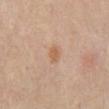Acquisition and patient details: A male patient aged 63 to 67. This image is a 15 mm lesion crop taken from a total-body photograph. On the abdomen. This is a white-light tile. The total-body-photography lesion software estimated a border-irregularity rating of about 2.5/10, a within-lesion color-variation index near 1.5/10, and radial color variation of about 0.5. The analysis additionally found a detector confidence of about 100 out of 100 that the crop contains a lesion.